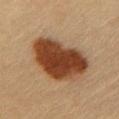No biopsy was performed on this lesion — it was imaged during a full skin examination and was not determined to be concerning. A female patient about 50 years old. A 15 mm close-up tile from a total-body photography series done for melanoma screening. From the chest.